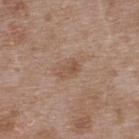Q: Is there a histopathology result?
A: catalogued during a skin exam; not biopsied
Q: How was the tile lit?
A: white-light
Q: Lesion location?
A: the upper back
Q: How large is the lesion?
A: ~2.5 mm (longest diameter)
Q: Automated lesion metrics?
A: an area of roughly 3.5 mm², a shape eccentricity near 0.75, and a shape-asymmetry score of about 0.15 (0 = symmetric); a classifier nevus-likeness of about 0/100 and a detector confidence of about 100 out of 100 that the crop contains a lesion
Q: What are the patient's age and sex?
A: male, approximately 50 years of age
Q: What kind of image is this?
A: ~15 mm tile from a whole-body skin photo